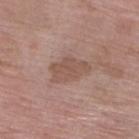This lesion was catalogued during total-body skin photography and was not selected for biopsy.